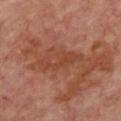Q: Was a biopsy performed?
A: imaged on a skin check; not biopsied
Q: How was this image acquired?
A: total-body-photography crop, ~15 mm field of view
Q: Lesion location?
A: the chest
Q: Who is the patient?
A: aged 53 to 57
Q: Automated lesion metrics?
A: a border-irregularity rating of about 8/10 and peripheral color asymmetry of about 0.5
Q: How was the tile lit?
A: cross-polarized
Q: What is the lesion's diameter?
A: ≈5.5 mm